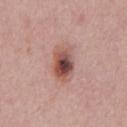Assessment:
Recorded during total-body skin imaging; not selected for excision or biopsy.
Acquisition and patient details:
A 15 mm close-up extracted from a 3D total-body photography capture. A male patient, in their 60s. On the abdomen.